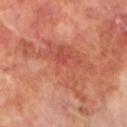The lesion was tiled from a total-body skin photograph and was not biopsied.
The lesion is on the left lower leg.
The subject is a male aged 68–72.
A roughly 15 mm field-of-view crop from a total-body skin photograph.